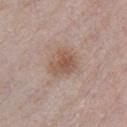biopsy status: imaged on a skin check; not biopsied
image: 15 mm crop, total-body photography
subject: male, about 30 years old